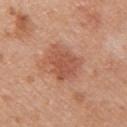The lesion was tiled from a total-body skin photograph and was not biopsied.
A region of skin cropped from a whole-body photographic capture, roughly 15 mm wide.
On the right upper arm.
A female subject in their 50s.